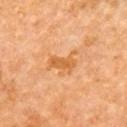{"biopsy_status": "not biopsied; imaged during a skin examination", "lighting": "cross-polarized", "site": "upper back", "lesion_size": {"long_diameter_mm_approx": 4.0}, "patient": {"sex": "female", "age_approx": 50}, "image": {"source": "total-body photography crop", "field_of_view_mm": 15}}